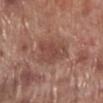Part of a total-body skin-imaging series; this lesion was reviewed on a skin check and was not flagged for biopsy.
On the right lower leg.
A region of skin cropped from a whole-body photographic capture, roughly 15 mm wide.
The total-body-photography lesion software estimated a lesion area of about 12 mm², a shape eccentricity near 0.7, and a symmetry-axis asymmetry near 0.25. The software also gave a lesion–skin lightness drop of about 8 and a normalized lesion–skin contrast near 6. The software also gave a classifier nevus-likeness of about 15/100 and a detector confidence of about 100 out of 100 that the crop contains a lesion.
A male patient roughly 70 years of age.
The tile uses white-light illumination.
The recorded lesion diameter is about 5 mm.